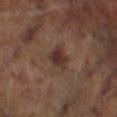Q: Is there a histopathology result?
A: total-body-photography surveillance lesion; no biopsy
Q: How was the tile lit?
A: cross-polarized illumination
Q: What did automated image analysis measure?
A: a footprint of about 6 mm², a shape eccentricity near 0.65, and two-axis asymmetry of about 0.3; a border-irregularity rating of about 3/10 and radial color variation of about 1
Q: What kind of image is this?
A: ~15 mm tile from a whole-body skin photo
Q: Lesion location?
A: the right lower leg
Q: What are the patient's age and sex?
A: male, aged approximately 70
Q: What is the lesion's diameter?
A: about 3 mm